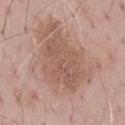Q: Is there a histopathology result?
A: imaged on a skin check; not biopsied
Q: What kind of image is this?
A: ~15 mm crop, total-body skin-cancer survey
Q: Patient demographics?
A: male, aged around 70
Q: Lesion location?
A: the mid back
Q: How was the tile lit?
A: white-light illumination
Q: Lesion size?
A: ~6.5 mm (longest diameter)